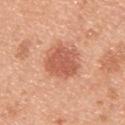biopsy status=imaged on a skin check; not biopsied
acquisition=~15 mm tile from a whole-body skin photo
image-analysis metrics=a mean CIELAB color near L≈58 a*≈28 b*≈34, a lesion–skin lightness drop of about 12, and a normalized border contrast of about 7.5
site=the back
patient=male, in their mid-40s